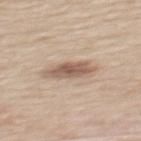Impression:
No biopsy was performed on this lesion — it was imaged during a full skin examination and was not determined to be concerning.
Clinical summary:
Approximately 5 mm at its widest. The total-body-photography lesion software estimated an area of roughly 9 mm², a shape eccentricity near 0.9, and a shape-asymmetry score of about 0.2 (0 = symmetric). It also reported a border-irregularity index near 2.5/10 and peripheral color asymmetry of about 1. The software also gave an automated nevus-likeness rating near 40 out of 100. Captured under white-light illumination. The lesion is on the back. The subject is a male about 60 years old. This image is a 15 mm lesion crop taken from a total-body photograph.